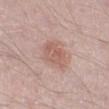Recorded during total-body skin imaging; not selected for excision or biopsy. The patient is a male roughly 50 years of age. Located on the right lower leg. A 15 mm close-up extracted from a 3D total-body photography capture.